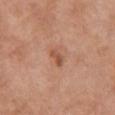biopsy status: imaged on a skin check; not biopsied
location: the front of the torso
image source: total-body-photography crop, ~15 mm field of view
diameter: about 2.5 mm
patient: female, in their mid- to late 60s